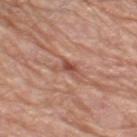<tbp_lesion>
<biopsy_status>not biopsied; imaged during a skin examination</biopsy_status>
<patient>
  <sex>male</sex>
  <age_approx>80</age_approx>
</patient>
<image>
  <source>total-body photography crop</source>
  <field_of_view_mm>15</field_of_view_mm>
</image>
<automated_metrics>
  <cielab_L>51</cielab_L>
  <cielab_a>25</cielab_a>
  <cielab_b>29</cielab_b>
  <vs_skin_darker_L>10.0</vs_skin_darker_L>
</automated_metrics>
<lighting>white-light</lighting>
<site>left thigh</site>
</tbp_lesion>